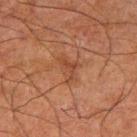notes: imaged on a skin check; not biopsied
acquisition: total-body-photography crop, ~15 mm field of view
anatomic site: the right upper arm
diameter: ≈3 mm
subject: male, approximately 70 years of age